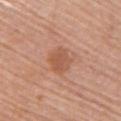Q: Was a biopsy performed?
A: total-body-photography surveillance lesion; no biopsy
Q: What is the imaging modality?
A: ~15 mm tile from a whole-body skin photo
Q: Lesion location?
A: the upper back
Q: Illumination type?
A: white-light illumination
Q: Patient demographics?
A: female, aged 63–67
Q: What is the lesion's diameter?
A: about 3 mm